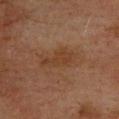Part of a total-body skin-imaging series; this lesion was reviewed on a skin check and was not flagged for biopsy.
A close-up tile cropped from a whole-body skin photograph, about 15 mm across.
The lesion is located on the back.
Measured at roughly 4.5 mm in maximum diameter.
A male subject, aged approximately 75.
Captured under cross-polarized illumination.
The total-body-photography lesion software estimated a lesion area of about 7.5 mm², an eccentricity of roughly 0.9, and two-axis asymmetry of about 0.3. The software also gave an average lesion color of about L≈32 a*≈17 b*≈27 (CIELAB) and roughly 5 lightness units darker than nearby skin.